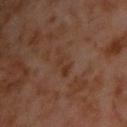Part of a total-body skin-imaging series; this lesion was reviewed on a skin check and was not flagged for biopsy. The lesion is on the chest. A male subject, aged approximately 60. The total-body-photography lesion software estimated a footprint of about 5 mm² and a shape-asymmetry score of about 0.35 (0 = symmetric). It also reported an average lesion color of about L≈32 a*≈18 b*≈26 (CIELAB), roughly 4 lightness units darker than nearby skin, and a normalized lesion–skin contrast near 5.5. And it measured a border-irregularity index near 4/10 and a within-lesion color-variation index near 2.5/10. This image is a 15 mm lesion crop taken from a total-body photograph. Imaged with cross-polarized lighting. Approximately 4 mm at its widest.